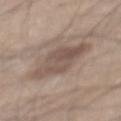| field | value |
|---|---|
| follow-up | catalogued during a skin exam; not biopsied |
| subject | male, aged 63–67 |
| body site | the mid back |
| image | ~15 mm tile from a whole-body skin photo |
| illumination | white-light |